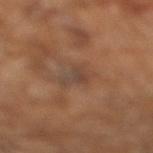{"biopsy_status": "not biopsied; imaged during a skin examination", "image": {"source": "total-body photography crop", "field_of_view_mm": 15}, "automated_metrics": {"area_mm2_approx": 5.0, "eccentricity": 0.8, "shape_asymmetry": 0.5, "border_irregularity_0_10": 5.0, "color_variation_0_10": 2.5}, "lesion_size": {"long_diameter_mm_approx": 3.5}, "lighting": "cross-polarized", "patient": {"sex": "male", "age_approx": 65}}